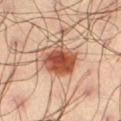| feature | finding |
|---|---|
| body site | the leg |
| TBP lesion metrics | an average lesion color of about L≈51 a*≈26 b*≈33 (CIELAB), a lesion–skin lightness drop of about 17, and a normalized lesion–skin contrast near 11.5; a border-irregularity rating of about 3/10, a color-variation rating of about 5.5/10, and a peripheral color-asymmetry measure near 1.5 |
| image source | total-body-photography crop, ~15 mm field of view |
| tile lighting | cross-polarized |
| size | ≈5 mm |
| patient | male, aged 38–42 |